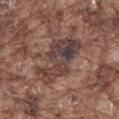| field | value |
|---|---|
| workup | no biopsy performed (imaged during a skin exam) |
| body site | the mid back |
| subject | male, aged approximately 75 |
| diameter | about 6.5 mm |
| imaging modality | ~15 mm tile from a whole-body skin photo |
| automated metrics | an area of roughly 20 mm² and two-axis asymmetry of about 0.35 |